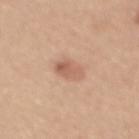Captured during whole-body skin photography for melanoma surveillance; the lesion was not biopsied. The patient is a female approximately 55 years of age. A 15 mm crop from a total-body photograph taken for skin-cancer surveillance. The lesion is on the mid back. Imaged with white-light lighting. The recorded lesion diameter is about 3.5 mm.